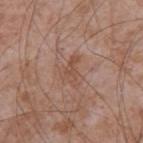Findings:
– patient — male, aged around 75
– size — ~3.5 mm (longest diameter)
– image — 15 mm crop, total-body photography
– body site — the left upper arm